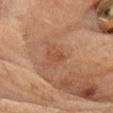biopsy status: total-body-photography surveillance lesion; no biopsy
image source: ~15 mm crop, total-body skin-cancer survey
location: the head or neck
subject: male, about 70 years old